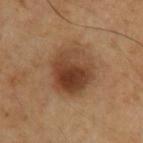Clinical impression: Imaged during a routine full-body skin examination; the lesion was not biopsied and no histopathology is available. Acquisition and patient details: A male subject, approximately 70 years of age. A close-up tile cropped from a whole-body skin photograph, about 15 mm across. Imaged with cross-polarized lighting. Located on the left upper arm.